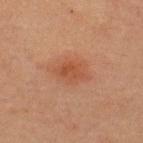follow-up = catalogued during a skin exam; not biopsied
image = ~15 mm tile from a whole-body skin photo
subject = male, approximately 60 years of age
anatomic site = the upper back